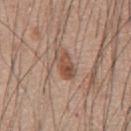{"biopsy_status": "not biopsied; imaged during a skin examination", "lesion_size": {"long_diameter_mm_approx": 3.5}, "automated_metrics": {"area_mm2_approx": 6.0, "eccentricity": 0.8, "shape_asymmetry": 0.25, "cielab_L": 51, "cielab_a": 20, "cielab_b": 28, "vs_skin_darker_L": 10.0, "vs_skin_contrast_norm": 7.5, "nevus_likeness_0_100": 85, "lesion_detection_confidence_0_100": 100}, "lighting": "white-light", "image": {"source": "total-body photography crop", "field_of_view_mm": 15}, "patient": {"sex": "male", "age_approx": 60}, "site": "chest"}